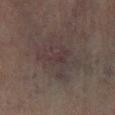No biopsy was performed on this lesion — it was imaged during a full skin examination and was not determined to be concerning. A lesion tile, about 15 mm wide, cut from a 3D total-body photograph. A female subject in their 80s. The tile uses cross-polarized illumination. On the left lower leg. The total-body-photography lesion software estimated a lesion area of about 19 mm² and a symmetry-axis asymmetry near 0.3. Measured at roughly 6 mm in maximum diameter.